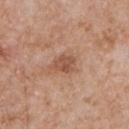{"biopsy_status": "not biopsied; imaged during a skin examination", "lesion_size": {"long_diameter_mm_approx": 3.0}, "patient": {"sex": "male", "age_approx": 65}, "site": "chest", "automated_metrics": {"area_mm2_approx": 5.5, "shape_asymmetry": 0.35, "cielab_L": 52, "cielab_a": 22, "cielab_b": 31, "vs_skin_contrast_norm": 6.5, "border_irregularity_0_10": 3.5, "color_variation_0_10": 2.0, "peripheral_color_asymmetry": 1.0, "nevus_likeness_0_100": 0, "lesion_detection_confidence_0_100": 100}, "image": {"source": "total-body photography crop", "field_of_view_mm": 15}, "lighting": "white-light"}